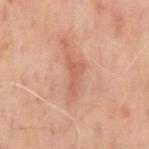Captured under cross-polarized illumination. This image is a 15 mm lesion crop taken from a total-body photograph. A male subject, about 65 years old. The lesion's longest dimension is about 4.5 mm. From the back.